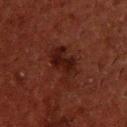follow-up: total-body-photography surveillance lesion; no biopsy
tile lighting: cross-polarized
image source: ~15 mm crop, total-body skin-cancer survey
patient: male, about 50 years old
anatomic site: the back